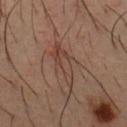notes: imaged on a skin check; not biopsied | lighting: cross-polarized illumination | TBP lesion metrics: an automated nevus-likeness rating near 0 out of 100 and a lesion-detection confidence of about 85/100 | site: the chest | imaging modality: ~15 mm crop, total-body skin-cancer survey | patient: male, in their mid-30s | diameter: ≈5.5 mm.